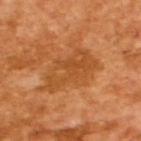workup=catalogued during a skin exam; not biopsied | subject=male, in their mid-60s | image=~15 mm tile from a whole-body skin photo | lesion diameter=~6.5 mm (longest diameter) | illumination=cross-polarized.